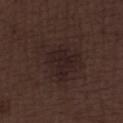Impression: Imaged during a routine full-body skin examination; the lesion was not biopsied and no histopathology is available. Context: A 15 mm close-up extracted from a 3D total-body photography capture. From the leg. A male subject, aged 68–72. About 6 mm across. Captured under white-light illumination.